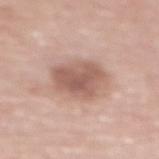The subject is a female roughly 65 years of age. A 15 mm close-up tile from a total-body photography series done for melanoma screening. Measured at roughly 4.5 mm in maximum diameter. Imaged with white-light lighting. On the chest. The lesion-visualizer software estimated a lesion area of about 15 mm², an outline eccentricity of about 0.5 (0 = round, 1 = elongated), and two-axis asymmetry of about 0.2. And it measured a border-irregularity rating of about 2/10, internal color variation of about 4 on a 0–10 scale, and radial color variation of about 1.5.